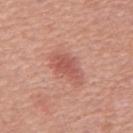Part of a total-body skin-imaging series; this lesion was reviewed on a skin check and was not flagged for biopsy. A lesion tile, about 15 mm wide, cut from a 3D total-body photograph. Automated tile analysis of the lesion measured a footprint of about 8 mm² and a shape-asymmetry score of about 0.3 (0 = symmetric). It also reported a lesion color around L≈55 a*≈28 b*≈28 in CIELAB, a lesion–skin lightness drop of about 9, and a normalized lesion–skin contrast near 6.5. The analysis additionally found an automated nevus-likeness rating near 80 out of 100 and a detector confidence of about 100 out of 100 that the crop contains a lesion. Located on the back. A male subject, in their mid- to late 60s. The lesion's longest dimension is about 4 mm.